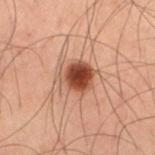Case summary:
* notes: total-body-photography surveillance lesion; no biopsy
* image source: 15 mm crop, total-body photography
* image-analysis metrics: an eccentricity of roughly 0.45; a border-irregularity index near 1.5/10, internal color variation of about 5.5 on a 0–10 scale, and radial color variation of about 1.5; a nevus-likeness score of about 100/100
* body site: the right thigh
* lesion size: ≈3.5 mm
* subject: male, in their 60s